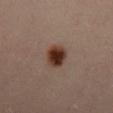{
  "lighting": "cross-polarized",
  "lesion_size": {
    "long_diameter_mm_approx": 3.5
  },
  "image": {
    "source": "total-body photography crop",
    "field_of_view_mm": 15
  },
  "automated_metrics": {
    "eccentricity": 0.7,
    "shape_asymmetry": 0.15,
    "cielab_L": 33,
    "cielab_a": 20,
    "cielab_b": 26,
    "vs_skin_contrast_norm": 13.5,
    "lesion_detection_confidence_0_100": 100
  },
  "patient": {
    "sex": "male",
    "age_approx": 35
  },
  "site": "mid back",
  "diagnosis": {
    "histopathology": "compound melanocytic nevus",
    "malignancy": "benign",
    "taxonomic_path": [
      "Benign",
      "Benign melanocytic proliferations",
      "Nevus",
      "Nevus, NOS, Compound"
    ]
  }
}